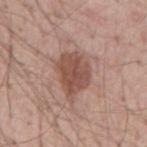Case summary:
– subject · male, approximately 55 years of age
– site · the mid back
– lighting · white-light illumination
– image · ~15 mm crop, total-body skin-cancer survey